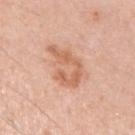follow-up: total-body-photography surveillance lesion; no biopsy
image: 15 mm crop, total-body photography
site: the left upper arm
lesion size: about 5 mm
illumination: white-light
subject: male, approximately 50 years of age